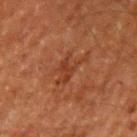Q: What are the patient's age and sex?
A: male, aged around 60
Q: Lesion location?
A: the left upper arm
Q: How was this image acquired?
A: ~15 mm tile from a whole-body skin photo
Q: How large is the lesion?
A: ≈4.5 mm
Q: What lighting was used for the tile?
A: cross-polarized illumination